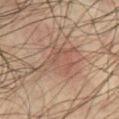Clinical impression:
Captured during whole-body skin photography for melanoma surveillance; the lesion was not biopsied.